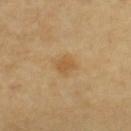Q: Automated lesion metrics?
A: an area of roughly 4.5 mm² and a symmetry-axis asymmetry near 0.35; a mean CIELAB color near L≈56 a*≈17 b*≈41; a border-irregularity index near 3/10, a color-variation rating of about 1.5/10, and radial color variation of about 0.5
Q: What is the imaging modality?
A: ~15 mm crop, total-body skin-cancer survey
Q: How large is the lesion?
A: about 2.5 mm
Q: What is the anatomic site?
A: the right upper arm
Q: What are the patient's age and sex?
A: female, aged around 70